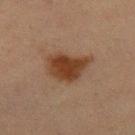Findings:
* biopsy status: catalogued during a skin exam; not biopsied
* subject: female, roughly 40 years of age
* location: the right thigh
* image: 15 mm crop, total-body photography